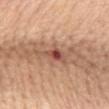biopsy_status: not biopsied; imaged during a skin examination
patient:
  sex: female
  age_approx: 65
image:
  source: total-body photography crop
  field_of_view_mm: 15
site: mid back